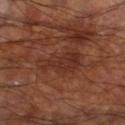{"biopsy_status": "not biopsied; imaged during a skin examination", "lesion_size": {"long_diameter_mm_approx": 5.5}, "image": {"source": "total-body photography crop", "field_of_view_mm": 15}, "site": "right lower leg", "patient": {"sex": "male", "age_approx": 60}, "lighting": "cross-polarized"}